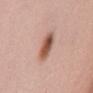Case summary:
– biopsy status: imaged on a skin check; not biopsied
– patient: female, aged 38 to 42
– location: the mid back
– diameter: ~3.5 mm (longest diameter)
– image source: 15 mm crop, total-body photography